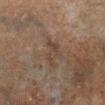| feature | finding |
|---|---|
| follow-up | no biopsy performed (imaged during a skin exam) |
| lighting | cross-polarized |
| size | ≈3.5 mm |
| image | ~15 mm tile from a whole-body skin photo |
| automated lesion analysis | a shape-asymmetry score of about 0.55 (0 = symmetric); a detector confidence of about 65 out of 100 that the crop contains a lesion |
| anatomic site | the right lower leg |
| patient | male, approximately 60 years of age |